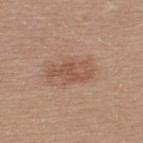Clinical summary:
A male subject, in their 40s. Approximately 5 mm at its widest. A lesion tile, about 15 mm wide, cut from a 3D total-body photograph. The tile uses white-light illumination. Automated image analysis of the tile measured an eccentricity of roughly 0.85 and a symmetry-axis asymmetry near 0.3. And it measured border irregularity of about 3.5 on a 0–10 scale and a color-variation rating of about 3.5/10. On the upper back.